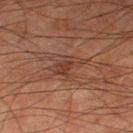Q: Was this lesion biopsied?
A: total-body-photography surveillance lesion; no biopsy
Q: Lesion size?
A: ~3.5 mm (longest diameter)
Q: What lighting was used for the tile?
A: cross-polarized
Q: Where on the body is the lesion?
A: the left thigh
Q: Who is the patient?
A: male, roughly 80 years of age
Q: How was this image acquired?
A: total-body-photography crop, ~15 mm field of view